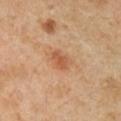Impression:
Part of a total-body skin-imaging series; this lesion was reviewed on a skin check and was not flagged for biopsy.
Background:
This is a cross-polarized tile. The subject is a female in their 40s. About 2.5 mm across. A close-up tile cropped from a whole-body skin photograph, about 15 mm across. From the left forearm.